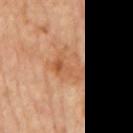{
  "biopsy_status": "not biopsied; imaged during a skin examination",
  "image": {
    "source": "total-body photography crop",
    "field_of_view_mm": 15
  },
  "site": "back",
  "lesion_size": {
    "long_diameter_mm_approx": 5.0
  },
  "lighting": "cross-polarized",
  "patient": {
    "sex": "male",
    "age_approx": 60
  }
}